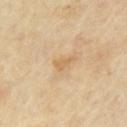Imaged during a routine full-body skin examination; the lesion was not biopsied and no histopathology is available. On the left upper arm. A male subject in their mid-60s. A region of skin cropped from a whole-body photographic capture, roughly 15 mm wide.